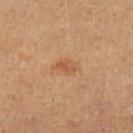Assessment: This lesion was catalogued during total-body skin photography and was not selected for biopsy. Clinical summary: Measured at roughly 3 mm in maximum diameter. The total-body-photography lesion software estimated a border-irregularity index near 3/10, a within-lesion color-variation index near 2/10, and a peripheral color-asymmetry measure near 0.5. It also reported a classifier nevus-likeness of about 5/100 and lesion-presence confidence of about 100/100. A 15 mm close-up extracted from a 3D total-body photography capture. The subject is male. From the left lower leg. Imaged with cross-polarized lighting.